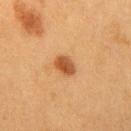{
  "site": "chest",
  "patient": {
    "sex": "female",
    "age_approx": 50
  },
  "image": {
    "source": "total-body photography crop",
    "field_of_view_mm": 15
  }
}